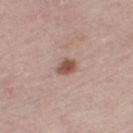Q: Was a biopsy performed?
A: no biopsy performed (imaged during a skin exam)
Q: Lesion location?
A: the leg
Q: Who is the patient?
A: female, aged approximately 65
Q: What kind of image is this?
A: 15 mm crop, total-body photography